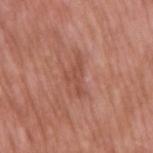Impression:
Imaged during a routine full-body skin examination; the lesion was not biopsied and no histopathology is available.
Context:
Cropped from a whole-body photographic skin survey; the tile spans about 15 mm. The total-body-photography lesion software estimated a color-variation rating of about 1.5/10 and radial color variation of about 0.5. The software also gave a nevus-likeness score of about 0/100 and a lesion-detection confidence of about 100/100. A male patient in their mid-50s. From the right upper arm. Measured at roughly 4 mm in maximum diameter. Imaged with white-light lighting.